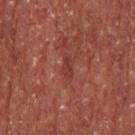No biopsy was performed on this lesion — it was imaged during a full skin examination and was not determined to be concerning. From the head or neck. This is a cross-polarized tile. Cropped from a whole-body photographic skin survey; the tile spans about 15 mm. The subject is a male approximately 55 years of age. Approximately 2.5 mm at its widest.